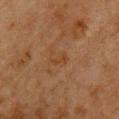notes=imaged on a skin check; not biopsied | patient=male, aged 58 to 62 | imaging modality=15 mm crop, total-body photography | anatomic site=the chest.